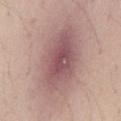workup — catalogued during a skin exam; not biopsied
location — the back
subject — male, aged around 35
lesion size — about 10 mm
illumination — white-light
imaging modality — 15 mm crop, total-body photography
TBP lesion metrics — a lesion area of about 41 mm² and an outline eccentricity of about 0.85 (0 = round, 1 = elongated); about 12 CIELAB-L* units darker than the surrounding skin and a normalized lesion–skin contrast near 8.5